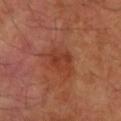{"biopsy_status": "not biopsied; imaged during a skin examination", "image": {"source": "total-body photography crop", "field_of_view_mm": 15}, "patient": {"sex": "male", "age_approx": 55}, "lesion_size": {"long_diameter_mm_approx": 4.0}, "site": "right forearm"}